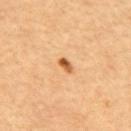The lesion was photographed on a routine skin check and not biopsied; there is no pathology result. A close-up tile cropped from a whole-body skin photograph, about 15 mm across. The recorded lesion diameter is about 2 mm. The lesion is located on the mid back. A male subject in their 60s. This is a cross-polarized tile.